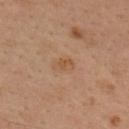Captured during whole-body skin photography for melanoma surveillance; the lesion was not biopsied. A 15 mm close-up extracted from a 3D total-body photography capture. On the upper back. The patient is a male roughly 35 years of age. The lesion's longest dimension is about 2.5 mm. The total-body-photography lesion software estimated a footprint of about 3.5 mm² and an outline eccentricity of about 0.8 (0 = round, 1 = elongated). And it measured a lesion color around L≈50 a*≈19 b*≈33 in CIELAB. It also reported border irregularity of about 2 on a 0–10 scale, internal color variation of about 2 on a 0–10 scale, and peripheral color asymmetry of about 1. The analysis additionally found an automated nevus-likeness rating near 5 out of 100 and a detector confidence of about 100 out of 100 that the crop contains a lesion.